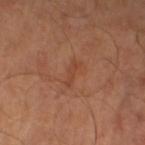Q: Is there a histopathology result?
A: imaged on a skin check; not biopsied